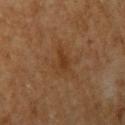Imaged during a routine full-body skin examination; the lesion was not biopsied and no histopathology is available. From the left upper arm. A region of skin cropped from a whole-body photographic capture, roughly 15 mm wide. The tile uses cross-polarized illumination. The recorded lesion diameter is about 3 mm. A male subject in their 60s. Automated image analysis of the tile measured an area of roughly 5 mm², a shape eccentricity near 0.65, and two-axis asymmetry of about 0.4.